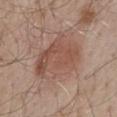Imaged during a routine full-body skin examination; the lesion was not biopsied and no histopathology is available. The subject is a male in their mid-50s. From the mid back. An algorithmic analysis of the crop reported a border-irregularity index near 2/10, a color-variation rating of about 4.5/10, and peripheral color asymmetry of about 1.5. It also reported a nevus-likeness score of about 85/100 and lesion-presence confidence of about 100/100. The recorded lesion diameter is about 6.5 mm. Captured under white-light illumination. Cropped from a whole-body photographic skin survey; the tile spans about 15 mm.